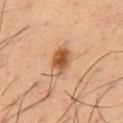biopsy status: catalogued during a skin exam; not biopsied | acquisition: total-body-photography crop, ~15 mm field of view | size: about 4 mm | automated lesion analysis: a footprint of about 8 mm², an outline eccentricity of about 0.75 (0 = round, 1 = elongated), and a symmetry-axis asymmetry near 0.15; border irregularity of about 1.5 on a 0–10 scale, a within-lesion color-variation index near 7.5/10, and radial color variation of about 2 | location: the chest | lighting: cross-polarized illumination | subject: male, aged around 35.